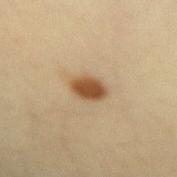A female patient, aged around 30.
Cropped from a whole-body photographic skin survey; the tile spans about 15 mm.
On the left forearm.
This is a cross-polarized tile.
Approximately 3.5 mm at its widest.